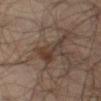Assessment: Captured during whole-body skin photography for melanoma surveillance; the lesion was not biopsied. Image and clinical context: A male subject in their mid- to late 60s. Approximately 5.5 mm at its widest. The lesion is located on the left thigh. This image is a 15 mm lesion crop taken from a total-body photograph. Imaged with cross-polarized lighting.